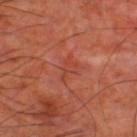<case>
  <biopsy_status>not biopsied; imaged during a skin examination</biopsy_status>
</case>